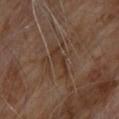  biopsy_status: not biopsied; imaged during a skin examination
  lesion_size:
    long_diameter_mm_approx: 4.0
  patient:
    sex: male
    age_approx: 70
  image:
    source: total-body photography crop
    field_of_view_mm: 15
  lighting: cross-polarized
  site: upper back